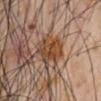Q: Was this lesion biopsied?
A: catalogued during a skin exam; not biopsied
Q: What are the patient's age and sex?
A: male, aged approximately 55
Q: Automated lesion metrics?
A: a lesion area of about 11 mm² and an outline eccentricity of about 0.7 (0 = round, 1 = elongated); a border-irregularity index near 3/10, a within-lesion color-variation index near 6.5/10, and radial color variation of about 2
Q: How was this image acquired?
A: ~15 mm crop, total-body skin-cancer survey
Q: Where on the body is the lesion?
A: the chest
Q: What is the lesion's diameter?
A: about 4.5 mm
Q: What lighting was used for the tile?
A: white-light illumination